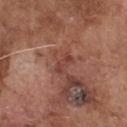tile lighting = white-light illumination; subject = male, aged 73 to 77; automated metrics = lesion-presence confidence of about 90/100; diameter = ~2.5 mm (longest diameter); acquisition = total-body-photography crop, ~15 mm field of view; body site = the chest.